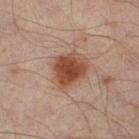workup: catalogued during a skin exam; not biopsied
acquisition: ~15 mm tile from a whole-body skin photo
lighting: cross-polarized
site: the right thigh
subject: male, in their mid-60s
image-analysis metrics: a shape-asymmetry score of about 0.25 (0 = symmetric); a lesion color around L≈45 a*≈21 b*≈29 in CIELAB, roughly 12 lightness units darker than nearby skin, and a normalized lesion–skin contrast near 10; a nevus-likeness score of about 100/100 and lesion-presence confidence of about 100/100
diameter: ~4.5 mm (longest diameter)